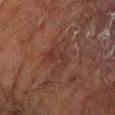The lesion was tiled from a total-body skin photograph and was not biopsied. About 3.5 mm across. The tile uses cross-polarized illumination. From the left forearm. The lesion-visualizer software estimated a lesion color around L≈25 a*≈17 b*≈19 in CIELAB, about 4 CIELAB-L* units darker than the surrounding skin, and a normalized lesion–skin contrast near 5. Cropped from a whole-body photographic skin survey; the tile spans about 15 mm. The subject is a male about 60 years old.